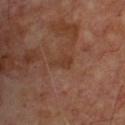Assessment: The lesion was tiled from a total-body skin photograph and was not biopsied. Clinical summary: A 15 mm crop from a total-body photograph taken for skin-cancer surveillance. The subject is a male in their mid- to late 60s. Imaged with cross-polarized lighting.